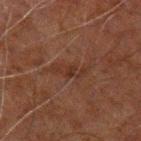Captured during whole-body skin photography for melanoma surveillance; the lesion was not biopsied.
Approximately 4.5 mm at its widest.
The tile uses cross-polarized illumination.
The lesion is on the left upper arm.
A male patient aged approximately 60.
A 15 mm close-up tile from a total-body photography series done for melanoma screening.